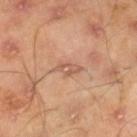Q: Was a biopsy performed?
A: catalogued during a skin exam; not biopsied
Q: Illumination type?
A: cross-polarized illumination
Q: What kind of image is this?
A: ~15 mm crop, total-body skin-cancer survey
Q: Lesion location?
A: the leg
Q: What is the lesion's diameter?
A: about 3 mm
Q: Who is the patient?
A: male, in their mid-40s